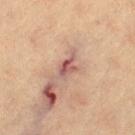{
  "biopsy_status": "not biopsied; imaged during a skin examination",
  "patient": {
    "sex": "female",
    "age_approx": 65
  },
  "site": "left thigh",
  "lesion_size": {
    "long_diameter_mm_approx": 3.5
  },
  "image": {
    "source": "total-body photography crop",
    "field_of_view_mm": 15
  },
  "lighting": "cross-polarized"
}